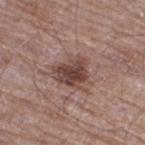The lesion was photographed on a routine skin check and not biopsied; there is no pathology result. From the right thigh. Automated image analysis of the tile measured a mean CIELAB color near L≈44 a*≈19 b*≈22 and a normalized lesion–skin contrast near 9. A male subject aged around 70. Imaged with white-light lighting. A roughly 15 mm field-of-view crop from a total-body skin photograph.